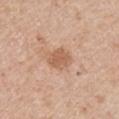Located on the right upper arm. A 15 mm close-up tile from a total-body photography series done for melanoma screening. The lesion's longest dimension is about 3 mm. The tile uses white-light illumination. The patient is a male aged approximately 70. An algorithmic analysis of the crop reported an average lesion color of about L≈59 a*≈21 b*≈33 (CIELAB), a lesion–skin lightness drop of about 9, and a lesion-to-skin contrast of about 6.5 (normalized; higher = more distinct). The software also gave border irregularity of about 1.5 on a 0–10 scale and internal color variation of about 2 on a 0–10 scale.